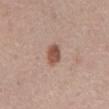notes: catalogued during a skin exam; not biopsied
image source: ~15 mm tile from a whole-body skin photo
lesion diameter: about 3 mm
lighting: white-light illumination
anatomic site: the abdomen
patient: male, aged 53–57